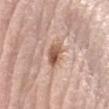Impression: Captured during whole-body skin photography for melanoma surveillance; the lesion was not biopsied.